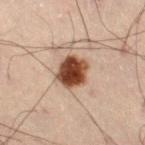notes: no biopsy performed (imaged during a skin exam)
imaging modality: ~15 mm tile from a whole-body skin photo
location: the right thigh
illumination: cross-polarized
TBP lesion metrics: a mean CIELAB color near L≈40 a*≈21 b*≈28, roughly 20 lightness units darker than nearby skin, and a lesion-to-skin contrast of about 15 (normalized; higher = more distinct)
subject: male, approximately 50 years of age
lesion size: about 3.5 mm